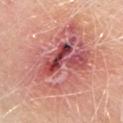Context: A male subject approximately 65 years of age. The tile uses white-light illumination. Approximately 10 mm at its widest. A 15 mm close-up tile from a total-body photography series done for melanoma screening. From the back.